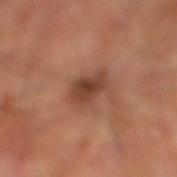• biopsy status — catalogued during a skin exam; not biopsied
• site — the leg
• patient — male, aged approximately 70
• imaging modality — ~15 mm crop, total-body skin-cancer survey